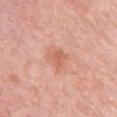- biopsy status: catalogued during a skin exam; not biopsied
- image: 15 mm crop, total-body photography
- lesion diameter: ≈2.5 mm
- body site: the left upper arm
- tile lighting: white-light
- TBP lesion metrics: an area of roughly 3.5 mm² and a shape-asymmetry score of about 0.45 (0 = symmetric)
- patient: female, roughly 65 years of age